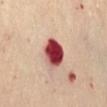Q: Is there a histopathology result?
A: no biopsy performed (imaged during a skin exam)
Q: What is the lesion's diameter?
A: ~4 mm (longest diameter)
Q: What did automated image analysis measure?
A: a lesion area of about 10 mm², a shape eccentricity near 0.5, and two-axis asymmetry of about 0.15; border irregularity of about 1.5 on a 0–10 scale and internal color variation of about 9.5 on a 0–10 scale
Q: Who is the patient?
A: female, roughly 60 years of age
Q: How was this image acquired?
A: ~15 mm tile from a whole-body skin photo
Q: Lesion location?
A: the abdomen
Q: What lighting was used for the tile?
A: cross-polarized